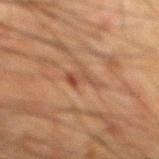Case summary:
- subject · male, approximately 65 years of age
- anatomic site · the back
- diameter · ~2.5 mm (longest diameter)
- imaging modality · 15 mm crop, total-body photography